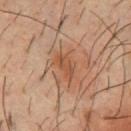Clinical impression: Part of a total-body skin-imaging series; this lesion was reviewed on a skin check and was not flagged for biopsy. Context: An algorithmic analysis of the crop reported a lesion area of about 4.5 mm², an eccentricity of roughly 0.9, and two-axis asymmetry of about 0.3. The analysis additionally found a border-irregularity index near 3.5/10, a color-variation rating of about 2.5/10, and radial color variation of about 1. It also reported a nevus-likeness score of about 0/100 and lesion-presence confidence of about 100/100. A 15 mm crop from a total-body photograph taken for skin-cancer surveillance. A male patient, aged 33 to 37. Imaged with cross-polarized lighting. From the chest. The lesion's longest dimension is about 3.5 mm.